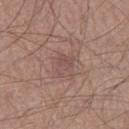• follow-up: no biopsy performed (imaged during a skin exam)
• body site: the left thigh
• image: ~15 mm tile from a whole-body skin photo
• patient: male, aged 23 to 27